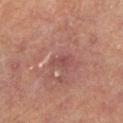biopsy status — total-body-photography surveillance lesion; no biopsy
image source — ~15 mm crop, total-body skin-cancer survey
site — the left lower leg
patient — male, in their mid-60s